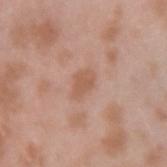Assessment: Imaged during a routine full-body skin examination; the lesion was not biopsied and no histopathology is available. Image and clinical context: Automated image analysis of the tile measured a lesion area of about 4.5 mm², an outline eccentricity of about 0.8 (0 = round, 1 = elongated), and two-axis asymmetry of about 0.3. And it measured an automated nevus-likeness rating near 0 out of 100 and a detector confidence of about 100 out of 100 that the crop contains a lesion. The lesion is on the left forearm. A close-up tile cropped from a whole-body skin photograph, about 15 mm across. The lesion's longest dimension is about 3 mm. The patient is a male aged around 40.